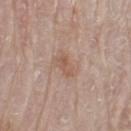Q: Was this lesion biopsied?
A: imaged on a skin check; not biopsied
Q: What is the imaging modality?
A: 15 mm crop, total-body photography
Q: What lighting was used for the tile?
A: white-light
Q: Where on the body is the lesion?
A: the left thigh
Q: What did automated image analysis measure?
A: roughly 7 lightness units darker than nearby skin and a normalized border contrast of about 5.5; a border-irregularity rating of about 2.5/10, a color-variation rating of about 3/10, and peripheral color asymmetry of about 1
Q: How large is the lesion?
A: ~2.5 mm (longest diameter)
Q: Who is the patient?
A: male, in their 80s